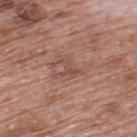Case summary:
– workup — imaged on a skin check; not biopsied
– patient — male, roughly 70 years of age
– body site — the upper back
– lesion size — about 2.5 mm
– image — ~15 mm tile from a whole-body skin photo
– illumination — white-light illumination